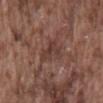The lesion was tiled from a total-body skin photograph and was not biopsied. The lesion-visualizer software estimated a mean CIELAB color near L≈38 a*≈20 b*≈23, about 7 CIELAB-L* units darker than the surrounding skin, and a lesion-to-skin contrast of about 6 (normalized; higher = more distinct). A region of skin cropped from a whole-body photographic capture, roughly 15 mm wide. Captured under white-light illumination. A male patient aged approximately 75. The lesion is on the mid back.